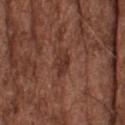biopsy status = imaged on a skin check; not biopsied
acquisition = 15 mm crop, total-body photography
illumination = white-light
image-analysis metrics = a border-irregularity index near 2/10, a color-variation rating of about 2/10, and peripheral color asymmetry of about 1
patient = male, aged around 75
location = the head or neck